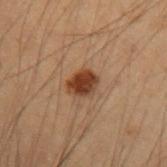* notes: catalogued during a skin exam; not biopsied
* TBP lesion metrics: a footprint of about 6 mm², an outline eccentricity of about 0.65 (0 = round, 1 = elongated), and two-axis asymmetry of about 0.15; a lesion color around L≈32 a*≈19 b*≈27 in CIELAB and a lesion-to-skin contrast of about 11.5 (normalized; higher = more distinct); a border-irregularity index near 1.5/10, a within-lesion color-variation index near 3.5/10, and peripheral color asymmetry of about 1; a lesion-detection confidence of about 100/100
* anatomic site: the arm
* subject: male, aged 53 to 57
* imaging modality: ~15 mm tile from a whole-body skin photo
* lesion size: about 3 mm